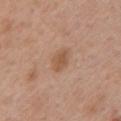The lesion was photographed on a routine skin check and not biopsied; there is no pathology result.
A female subject aged approximately 40.
A roughly 15 mm field-of-view crop from a total-body skin photograph.
On the left upper arm.
The lesion-visualizer software estimated a lesion area of about 4.5 mm², an outline eccentricity of about 0.8 (0 = round, 1 = elongated), and a shape-asymmetry score of about 0.15 (0 = symmetric). The software also gave a mean CIELAB color near L≈54 a*≈20 b*≈32, roughly 9 lightness units darker than nearby skin, and a normalized lesion–skin contrast near 6.5. The analysis additionally found a within-lesion color-variation index near 2.5/10 and a peripheral color-asymmetry measure near 1. And it measured an automated nevus-likeness rating near 55 out of 100 and lesion-presence confidence of about 100/100.
Approximately 3 mm at its widest.